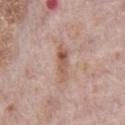No biopsy was performed on this lesion — it was imaged during a full skin examination and was not determined to be concerning. A male subject roughly 70 years of age. A lesion tile, about 15 mm wide, cut from a 3D total-body photograph. From the chest. The lesion's longest dimension is about 4.5 mm.